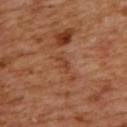This lesion was catalogued during total-body skin photography and was not selected for biopsy. The lesion is located on the upper back. A 15 mm close-up extracted from a 3D total-body photography capture. A female patient aged approximately 55. This is a cross-polarized tile. Automated image analysis of the tile measured a footprint of about 2.5 mm², an outline eccentricity of about 0.9 (0 = round, 1 = elongated), and a shape-asymmetry score of about 0.35 (0 = symmetric). And it measured a lesion color around L≈44 a*≈25 b*≈34 in CIELAB, about 6 CIELAB-L* units darker than the surrounding skin, and a lesion-to-skin contrast of about 5 (normalized; higher = more distinct). The analysis additionally found a color-variation rating of about 0/10 and peripheral color asymmetry of about 0.